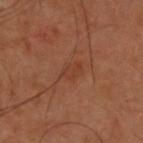notes — catalogued during a skin exam; not biopsied
subject — male, about 55 years old
imaging modality — total-body-photography crop, ~15 mm field of view
location — the back
lighting — cross-polarized